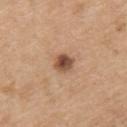– workup · catalogued during a skin exam; not biopsied
– imaging modality · 15 mm crop, total-body photography
– image-analysis metrics · a detector confidence of about 100 out of 100 that the crop contains a lesion
– lesion size · ~2.5 mm (longest diameter)
– anatomic site · the upper back
– subject · male, aged 68 to 72